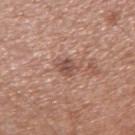Impression: Imaged during a routine full-body skin examination; the lesion was not biopsied and no histopathology is available. Acquisition and patient details: An algorithmic analysis of the crop reported a nevus-likeness score of about 0/100 and lesion-presence confidence of about 100/100. Captured under white-light illumination. A 15 mm crop from a total-body photograph taken for skin-cancer surveillance. A male subject, aged 53–57. Located on the right upper arm.